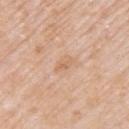{"biopsy_status": "not biopsied; imaged during a skin examination", "lesion_size": {"long_diameter_mm_approx": 3.0}, "site": "left upper arm", "lighting": "white-light", "image": {"source": "total-body photography crop", "field_of_view_mm": 15}, "patient": {"sex": "female", "age_approx": 60}, "automated_metrics": {"eccentricity": 0.85, "shape_asymmetry": 0.3, "border_irregularity_0_10": 3.0, "color_variation_0_10": 2.5, "peripheral_color_asymmetry": 1.0}}